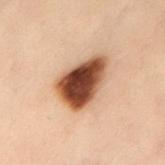Notes:
– workup: catalogued during a skin exam; not biopsied
– body site: the back
– tile lighting: cross-polarized illumination
– imaging modality: ~15 mm tile from a whole-body skin photo
– patient: female, aged 58–62